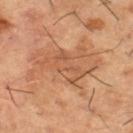{"biopsy_status": "not biopsied; imaged during a skin examination", "lighting": "cross-polarized", "image": {"source": "total-body photography crop", "field_of_view_mm": 15}, "lesion_size": {"long_diameter_mm_approx": 6.5}, "patient": {"sex": "male", "age_approx": 65}, "site": "right thigh"}